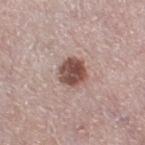Captured during whole-body skin photography for melanoma surveillance; the lesion was not biopsied. A roughly 15 mm field-of-view crop from a total-body skin photograph. Automated image analysis of the tile measured border irregularity of about 2 on a 0–10 scale, a within-lesion color-variation index near 4.5/10, and radial color variation of about 1.5. It also reported lesion-presence confidence of about 100/100. On the right thigh. Imaged with white-light lighting. A female subject, aged around 65. Longest diameter approximately 3 mm.